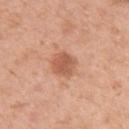This lesion was catalogued during total-body skin photography and was not selected for biopsy. A 15 mm crop from a total-body photograph taken for skin-cancer surveillance. Imaged with white-light lighting. The lesion is on the left upper arm. An algorithmic analysis of the crop reported a nevus-likeness score of about 75/100 and lesion-presence confidence of about 100/100. The patient is a female aged approximately 45. Measured at roughly 3 mm in maximum diameter.